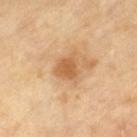Assessment:
The lesion was tiled from a total-body skin photograph and was not biopsied.
Acquisition and patient details:
The total-body-photography lesion software estimated a footprint of about 6.5 mm² and a shape-asymmetry score of about 0.35 (0 = symmetric). Longest diameter approximately 3 mm. A close-up tile cropped from a whole-body skin photograph, about 15 mm across. Imaged with cross-polarized lighting. The subject is a male in their mid- to late 60s.